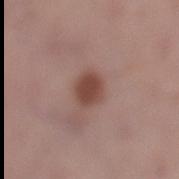Impression: Part of a total-body skin-imaging series; this lesion was reviewed on a skin check and was not flagged for biopsy. Background: Imaged with white-light lighting. A female subject roughly 50 years of age. This image is a 15 mm lesion crop taken from a total-body photograph. The lesion is on the left lower leg. The recorded lesion diameter is about 3 mm.